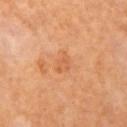Impression: Imaged during a routine full-body skin examination; the lesion was not biopsied and no histopathology is available. Acquisition and patient details: Imaged with cross-polarized lighting. About 3 mm across. A female subject, in their mid-60s. Automated tile analysis of the lesion measured a classifier nevus-likeness of about 0/100 and a lesion-detection confidence of about 100/100. Located on the arm. Cropped from a whole-body photographic skin survey; the tile spans about 15 mm.